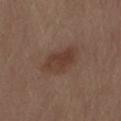location — the back
diameter — about 5 mm
patient — male, aged 68 to 72
automated metrics — an area of roughly 12 mm², an eccentricity of roughly 0.75, and a shape-asymmetry score of about 0.25 (0 = symmetric); about 7 CIELAB-L* units darker than the surrounding skin and a normalized lesion–skin contrast near 6.5; border irregularity of about 2.5 on a 0–10 scale, a within-lesion color-variation index near 3/10, and radial color variation of about 1; a detector confidence of about 100 out of 100 that the crop contains a lesion
tile lighting — white-light illumination
image — total-body-photography crop, ~15 mm field of view